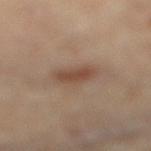| feature | finding |
|---|---|
| workup | total-body-photography surveillance lesion; no biopsy |
| automated lesion analysis | a lesion–skin lightness drop of about 9 and a normalized border contrast of about 7.5; a border-irregularity rating of about 2.5/10, a within-lesion color-variation index near 2/10, and peripheral color asymmetry of about 0.5; a nevus-likeness score of about 85/100 and a detector confidence of about 100 out of 100 that the crop contains a lesion |
| subject | female, in their 60s |
| site | the right lower leg |
| lesion size | about 3.5 mm |
| image source | ~15 mm tile from a whole-body skin photo |